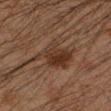Impression:
Recorded during total-body skin imaging; not selected for excision or biopsy.
Acquisition and patient details:
Automated image analysis of the tile measured an eccentricity of roughly 0.75 and a symmetry-axis asymmetry near 0.35. It also reported a mean CIELAB color near L≈25 a*≈14 b*≈21, a lesion–skin lightness drop of about 7, and a normalized lesion–skin contrast near 7.5. It also reported a border-irregularity rating of about 5.5/10, a within-lesion color-variation index near 4.5/10, and peripheral color asymmetry of about 1.5. It also reported an automated nevus-likeness rating near 60 out of 100 and a lesion-detection confidence of about 100/100. The recorded lesion diameter is about 6 mm. This is a cross-polarized tile. A male subject about 35 years old. The lesion is located on the arm. Cropped from a total-body skin-imaging series; the visible field is about 15 mm.